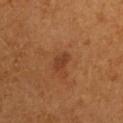Findings:
• notes: total-body-photography surveillance lesion; no biopsy
• lesion diameter: about 2.5 mm
• subject: male, roughly 55 years of age
• lighting: cross-polarized
• acquisition: ~15 mm crop, total-body skin-cancer survey
• anatomic site: the left upper arm
• automated metrics: a border-irregularity index near 2.5/10 and a within-lesion color-variation index near 1/10